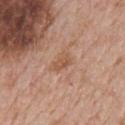notes: total-body-photography surveillance lesion; no biopsy
acquisition: ~15 mm crop, total-body skin-cancer survey
location: the chest
subject: male, approximately 75 years of age
lesion diameter: about 3 mm